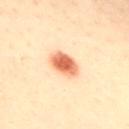Assessment:
Recorded during total-body skin imaging; not selected for excision or biopsy.
Acquisition and patient details:
Longest diameter approximately 3.5 mm. The lesion is located on the front of the torso. Imaged with cross-polarized lighting. The lesion-visualizer software estimated a footprint of about 7.5 mm², an outline eccentricity of about 0.65 (0 = round, 1 = elongated), and a shape-asymmetry score of about 0.15 (0 = symmetric). And it measured about 16 CIELAB-L* units darker than the surrounding skin and a normalized lesion–skin contrast near 10. It also reported a nevus-likeness score of about 100/100 and a detector confidence of about 100 out of 100 that the crop contains a lesion. The subject is a female aged 38–42. Cropped from a total-body skin-imaging series; the visible field is about 15 mm.